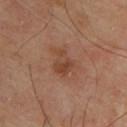follow-up: no biopsy performed (imaged during a skin exam) | patient: male, roughly 65 years of age | anatomic site: the upper back | acquisition: ~15 mm tile from a whole-body skin photo.